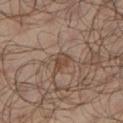Impression:
Captured during whole-body skin photography for melanoma surveillance; the lesion was not biopsied.
Context:
Approximately 3 mm at its widest. A lesion tile, about 15 mm wide, cut from a 3D total-body photograph. The patient is a male in their mid- to late 60s. The lesion is located on the left lower leg. The tile uses cross-polarized illumination.